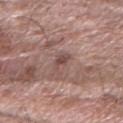Q: Was this lesion biopsied?
A: no biopsy performed (imaged during a skin exam)
Q: How was the tile lit?
A: white-light
Q: Patient demographics?
A: male, roughly 60 years of age
Q: How was this image acquired?
A: ~15 mm tile from a whole-body skin photo
Q: What did automated image analysis measure?
A: a lesion area of about 3.5 mm² and a symmetry-axis asymmetry near 0.4; a lesion color around L≈48 a*≈19 b*≈21 in CIELAB and roughly 9 lightness units darker than nearby skin; a within-lesion color-variation index near 3/10 and a peripheral color-asymmetry measure near 1; a classifier nevus-likeness of about 0/100 and a detector confidence of about 95 out of 100 that the crop contains a lesion
Q: Where on the body is the lesion?
A: the arm